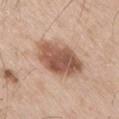No biopsy was performed on this lesion — it was imaged during a full skin examination and was not determined to be concerning. Imaged with white-light lighting. From the right thigh. The total-body-photography lesion software estimated a lesion color around L≈55 a*≈21 b*≈29 in CIELAB, roughly 15 lightness units darker than nearby skin, and a lesion-to-skin contrast of about 9.5 (normalized; higher = more distinct). The software also gave a border-irregularity rating of about 1.5/10, a within-lesion color-variation index near 5/10, and a peripheral color-asymmetry measure near 2. The software also gave an automated nevus-likeness rating near 85 out of 100 and lesion-presence confidence of about 100/100. A male patient in their 60s. A 15 mm close-up tile from a total-body photography series done for melanoma screening. About 6 mm across.